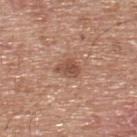Q: Is there a histopathology result?
A: imaged on a skin check; not biopsied
Q: How was this image acquired?
A: ~15 mm tile from a whole-body skin photo
Q: Who is the patient?
A: male, aged 63 to 67
Q: Illumination type?
A: white-light
Q: Lesion size?
A: ~2.5 mm (longest diameter)
Q: Automated lesion metrics?
A: an automated nevus-likeness rating near 55 out of 100 and a lesion-detection confidence of about 100/100
Q: Where on the body is the lesion?
A: the upper back